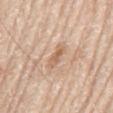Background:
The recorded lesion diameter is about 3 mm. The lesion is located on the mid back. This image is a 15 mm lesion crop taken from a total-body photograph. Imaged with white-light lighting. A male patient, roughly 80 years of age.